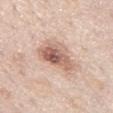Impression: Imaged during a routine full-body skin examination; the lesion was not biopsied and no histopathology is available. Clinical summary: The patient is a male aged 73–77. This is a white-light tile. Approximately 5.5 mm at its widest. A 15 mm crop from a total-body photograph taken for skin-cancer surveillance. From the chest.